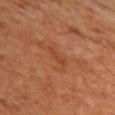Background: Located on the chest. A female subject aged around 55. The lesion's longest dimension is about 3 mm. Captured under cross-polarized illumination. A 15 mm close-up tile from a total-body photography series done for melanoma screening.